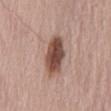| key | value |
|---|---|
| biopsy status | total-body-photography surveillance lesion; no biopsy |
| automated lesion analysis | a lesion area of about 12 mm² and an eccentricity of roughly 0.85; a border-irregularity rating of about 1.5/10, internal color variation of about 5.5 on a 0–10 scale, and peripheral color asymmetry of about 1.5; a nevus-likeness score of about 90/100 and lesion-presence confidence of about 100/100 |
| image | ~15 mm tile from a whole-body skin photo |
| diameter | ≈5.5 mm |
| body site | the back |
| patient | male, about 65 years old |